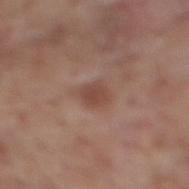follow-up: no biopsy performed (imaged during a skin exam)
lesion diameter: ≈2.5 mm
site: the mid back
automated metrics: a lesion area of about 4.5 mm², an eccentricity of roughly 0.65, and a symmetry-axis asymmetry near 0.3; a lesion–skin lightness drop of about 8 and a normalized border contrast of about 6.5; border irregularity of about 2.5 on a 0–10 scale and a within-lesion color-variation index near 2/10; a classifier nevus-likeness of about 20/100
illumination: white-light illumination
imaging modality: 15 mm crop, total-body photography
subject: male, about 60 years old